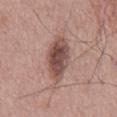Recorded during total-body skin imaging; not selected for excision or biopsy.
The lesion's longest dimension is about 6.5 mm.
A lesion tile, about 15 mm wide, cut from a 3D total-body photograph.
A male patient in their mid- to late 50s.
Automated tile analysis of the lesion measured a lesion area of about 14 mm². And it measured an average lesion color of about L≈49 a*≈20 b*≈23 (CIELAB), a lesion–skin lightness drop of about 14, and a lesion-to-skin contrast of about 9.5 (normalized; higher = more distinct). It also reported border irregularity of about 2.5 on a 0–10 scale, a within-lesion color-variation index near 5.5/10, and a peripheral color-asymmetry measure near 1.5. The analysis additionally found an automated nevus-likeness rating near 95 out of 100 and a detector confidence of about 100 out of 100 that the crop contains a lesion.
The tile uses white-light illumination.
The lesion is located on the mid back.